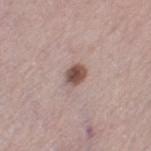Q: Is there a histopathology result?
A: total-body-photography surveillance lesion; no biopsy
Q: How large is the lesion?
A: ≈2.5 mm
Q: Patient demographics?
A: female, in their mid- to late 60s
Q: What lighting was used for the tile?
A: white-light illumination
Q: What is the anatomic site?
A: the leg
Q: What kind of image is this?
A: 15 mm crop, total-body photography